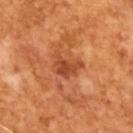Clinical impression: Part of a total-body skin-imaging series; this lesion was reviewed on a skin check and was not flagged for biopsy. Context: Longest diameter approximately 3.5 mm. The subject is a male in their mid-60s. The tile uses cross-polarized illumination. A 15 mm close-up extracted from a 3D total-body photography capture.